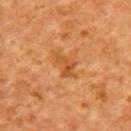Findings:
– workup · total-body-photography surveillance lesion; no biopsy
– tile lighting · cross-polarized illumination
– imaging modality · ~15 mm crop, total-body skin-cancer survey
– site · the upper back
– patient · female, about 40 years old
– lesion size · ~3.5 mm (longest diameter)